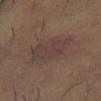Findings:
• acquisition · total-body-photography crop, ~15 mm field of view
• lesion diameter · about 8.5 mm
• patient · male, aged around 50
• tile lighting · cross-polarized
• location · the right thigh